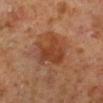{"biopsy_status": "not biopsied; imaged during a skin examination", "image": {"source": "total-body photography crop", "field_of_view_mm": 15}, "automated_metrics": {"border_irregularity_0_10": 4.0, "color_variation_0_10": 3.0, "peripheral_color_asymmetry": 1.0, "nevus_likeness_0_100": 60, "lesion_detection_confidence_0_100": 100}, "patient": {"sex": "male", "age_approx": 60}, "lesion_size": {"long_diameter_mm_approx": 4.5}, "site": "left lower leg"}